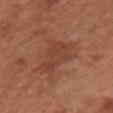Impression:
The lesion was tiled from a total-body skin photograph and was not biopsied.
Image and clinical context:
About 5 mm across. The subject is a female aged approximately 65. Cropped from a total-body skin-imaging series; the visible field is about 15 mm. On the chest. The total-body-photography lesion software estimated an average lesion color of about L≈42 a*≈24 b*≈30 (CIELAB), roughly 7 lightness units darker than nearby skin, and a normalized lesion–skin contrast near 5.5. And it measured border irregularity of about 5 on a 0–10 scale. And it measured a classifier nevus-likeness of about 0/100.